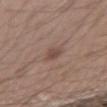follow-up — total-body-photography surveillance lesion; no biopsy
subject — male, aged 28 to 32
body site — the right forearm
image — 15 mm crop, total-body photography
lesion diameter — ≈2.5 mm
image-analysis metrics — a lesion area of about 3 mm², an outline eccentricity of about 0.85 (0 = round, 1 = elongated), and a symmetry-axis asymmetry near 0.4; border irregularity of about 3.5 on a 0–10 scale, a color-variation rating of about 1/10, and peripheral color asymmetry of about 0.5; an automated nevus-likeness rating near 20 out of 100 and lesion-presence confidence of about 100/100